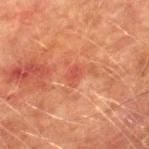• follow-up — no biopsy performed (imaged during a skin exam)